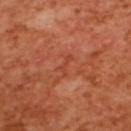Impression: The lesion was tiled from a total-body skin photograph and was not biopsied. Clinical summary: Approximately 2.5 mm at its widest. The subject is a female in their mid- to late 50s. Located on the upper back. Cropped from a total-body skin-imaging series; the visible field is about 15 mm. Imaged with cross-polarized lighting.